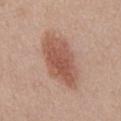Part of a total-body skin-imaging series; this lesion was reviewed on a skin check and was not flagged for biopsy. The lesion is located on the abdomen. A male subject aged approximately 60. The recorded lesion diameter is about 7.5 mm. The total-body-photography lesion software estimated a mean CIELAB color near L≈54 a*≈22 b*≈28. Cropped from a whole-body photographic skin survey; the tile spans about 15 mm.